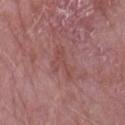Clinical impression:
Part of a total-body skin-imaging series; this lesion was reviewed on a skin check and was not flagged for biopsy.
Clinical summary:
The lesion-visualizer software estimated an area of roughly 6.5 mm². It also reported a lesion color around L≈48 a*≈24 b*≈23 in CIELAB, a lesion–skin lightness drop of about 6, and a lesion-to-skin contrast of about 5 (normalized; higher = more distinct). The analysis additionally found a border-irregularity rating of about 8/10. The lesion is located on the right forearm. The lesion's longest dimension is about 4.5 mm. A male patient, aged around 75. Imaged with white-light lighting. A 15 mm crop from a total-body photograph taken for skin-cancer surveillance.